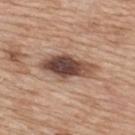Part of a total-body skin-imaging series; this lesion was reviewed on a skin check and was not flagged for biopsy. From the upper back. A 15 mm close-up tile from a total-body photography series done for melanoma screening. A female patient approximately 40 years of age. Longest diameter approximately 6.5 mm. The lesion-visualizer software estimated a footprint of about 14 mm², an outline eccentricity of about 0.9 (0 = round, 1 = elongated), and a shape-asymmetry score of about 0.2 (0 = symmetric). The software also gave a mean CIELAB color near L≈47 a*≈19 b*≈25. The software also gave a nevus-likeness score of about 90/100 and a lesion-detection confidence of about 100/100.